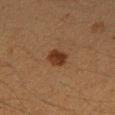follow-up: catalogued during a skin exam; not biopsied | body site: the right forearm | subject: female, aged 38–42 | image source: ~15 mm tile from a whole-body skin photo.